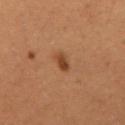Background: From the abdomen. Longest diameter approximately 2.5 mm. The patient is a male roughly 50 years of age. Cropped from a total-body skin-imaging series; the visible field is about 15 mm.